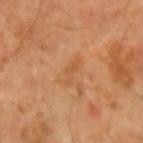Case summary:
- workup · imaged on a skin check; not biopsied
- automated metrics · a shape eccentricity near 0.9; a border-irregularity rating of about 6.5/10 and radial color variation of about 0
- imaging modality · ~15 mm crop, total-body skin-cancer survey
- lesion size · ≈3 mm
- anatomic site · the left forearm
- tile lighting · cross-polarized illumination
- patient · male, aged 63–67